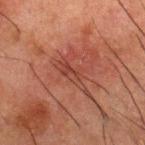Part of a total-body skin-imaging series; this lesion was reviewed on a skin check and was not flagged for biopsy. A male subject approximately 50 years of age. This is a cross-polarized tile. Automated tile analysis of the lesion measured a lesion area of about 7 mm², an eccentricity of roughly 0.9, and a symmetry-axis asymmetry near 0.6. It also reported a mean CIELAB color near L≈33 a*≈22 b*≈24, roughly 6 lightness units darker than nearby skin, and a normalized border contrast of about 5.5. Cropped from a whole-body photographic skin survey; the tile spans about 15 mm. Located on the upper back.